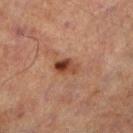biopsy_status: not biopsied; imaged during a skin examination
site: left lower leg
patient:
  sex: male
  age_approx: 70
image:
  source: total-body photography crop
  field_of_view_mm: 15
lesion_size:
  long_diameter_mm_approx: 3.0